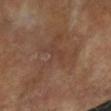Assessment:
No biopsy was performed on this lesion — it was imaged during a full skin examination and was not determined to be concerning.
Background:
A female patient aged approximately 75. The lesion is located on the arm. A close-up tile cropped from a whole-body skin photograph, about 15 mm across. This is a cross-polarized tile. The total-body-photography lesion software estimated a lesion area of about 22 mm², a shape eccentricity near 0.5, and a symmetry-axis asymmetry near 0.7. The analysis additionally found a mean CIELAB color near L≈41 a*≈18 b*≈27. The analysis additionally found a nevus-likeness score of about 0/100 and a lesion-detection confidence of about 95/100. Approximately 7.5 mm at its widest.